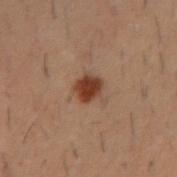workup = catalogued during a skin exam; not biopsied | anatomic site = the right forearm | image source = ~15 mm tile from a whole-body skin photo | subject = male, aged 28–32 | tile lighting = cross-polarized | size = ≈2.5 mm.